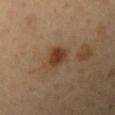{
  "biopsy_status": "not biopsied; imaged during a skin examination",
  "site": "left upper arm",
  "lesion_size": {
    "long_diameter_mm_approx": 3.0
  },
  "image": {
    "source": "total-body photography crop",
    "field_of_view_mm": 15
  },
  "patient": {
    "sex": "female",
    "age_approx": 50
  }
}